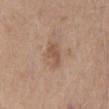biopsy status: no biopsy performed (imaged during a skin exam)
imaging modality: ~15 mm crop, total-body skin-cancer survey
illumination: white-light
location: the chest
patient: male, roughly 75 years of age
image-analysis metrics: an area of roughly 4.5 mm², an outline eccentricity of about 0.8 (0 = round, 1 = elongated), and a shape-asymmetry score of about 0.4 (0 = symmetric); border irregularity of about 4 on a 0–10 scale and radial color variation of about 0.5; an automated nevus-likeness rating near 0 out of 100 and lesion-presence confidence of about 100/100
lesion size: ≈3 mm